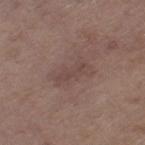Recorded during total-body skin imaging; not selected for excision or biopsy.